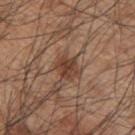A male subject, roughly 45 years of age. A region of skin cropped from a whole-body photographic capture, roughly 15 mm wide. Approximately 2.5 mm at its widest. Imaged with white-light lighting. On the upper back.